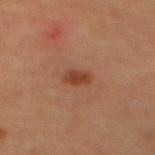Q: Was a biopsy performed?
A: total-body-photography surveillance lesion; no biopsy
Q: Where on the body is the lesion?
A: the mid back
Q: Patient demographics?
A: female
Q: How was this image acquired?
A: 15 mm crop, total-body photography